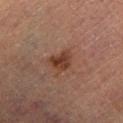The lesion was tiled from a total-body skin photograph and was not biopsied. Cropped from a whole-body photographic skin survey; the tile spans about 15 mm. Imaged with cross-polarized lighting. The subject is a male in their mid- to late 80s or older. On the left lower leg.